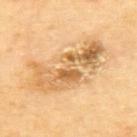The lesion was photographed on a routine skin check and not biopsied; there is no pathology result.
Captured under cross-polarized illumination.
About 8.5 mm across.
Located on the upper back.
The subject is a female aged 68 to 72.
Cropped from a whole-body photographic skin survey; the tile spans about 15 mm.
An algorithmic analysis of the crop reported an area of roughly 22 mm². The software also gave a mean CIELAB color near L≈67 a*≈20 b*≈45, a lesion–skin lightness drop of about 13, and a lesion-to-skin contrast of about 7.5 (normalized; higher = more distinct). The software also gave a nevus-likeness score of about 5/100 and a lesion-detection confidence of about 100/100.